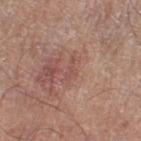{
  "biopsy_status": "not biopsied; imaged during a skin examination",
  "image": {
    "source": "total-body photography crop",
    "field_of_view_mm": 15
  },
  "lesion_size": {
    "long_diameter_mm_approx": 2.5
  },
  "patient": {
    "sex": "female",
    "age_approx": 80
  },
  "site": "right thigh",
  "lighting": "white-light",
  "automated_metrics": {
    "area_mm2_approx": 2.0,
    "eccentricity": 0.95,
    "shape_asymmetry": 0.45,
    "border_irregularity_0_10": 5.5,
    "color_variation_0_10": 0.0,
    "peripheral_color_asymmetry": 0.0,
    "nevus_likeness_0_100": 0,
    "lesion_detection_confidence_0_100": 95
  }
}